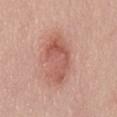Impression:
This lesion was catalogued during total-body skin photography and was not selected for biopsy.
Context:
A female patient, roughly 65 years of age. This image is a 15 mm lesion crop taken from a total-body photograph. The lesion is on the abdomen.